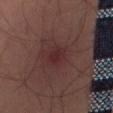A male subject, roughly 50 years of age. The lesion is located on the right thigh. A close-up tile cropped from a whole-body skin photograph, about 15 mm across.